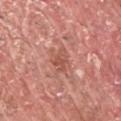Impression:
This lesion was catalogued during total-body skin photography and was not selected for biopsy.
Acquisition and patient details:
On the arm. The lesion's longest dimension is about 3 mm. An algorithmic analysis of the crop reported peripheral color asymmetry of about 1. The analysis additionally found an automated nevus-likeness rating near 0 out of 100 and lesion-presence confidence of about 100/100. The tile uses white-light illumination. A male patient aged around 40. A 15 mm crop from a total-body photograph taken for skin-cancer surveillance.